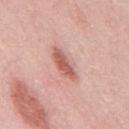<record>
  <patient>
    <sex>male</sex>
    <age_approx>55</age_approx>
  </patient>
  <image>
    <source>total-body photography crop</source>
    <field_of_view_mm>15</field_of_view_mm>
  </image>
  <lesion_size>
    <long_diameter_mm_approx>4.5</long_diameter_mm_approx>
  </lesion_size>
  <lighting>white-light</lighting>
  <automated_metrics>
    <area_mm2_approx>6.5</area_mm2_approx>
    <shape_asymmetry>0.2</shape_asymmetry>
    <nevus_likeness_0_100>90</nevus_likeness_0_100>
  </automated_metrics>
  <site>mid back</site>
</record>